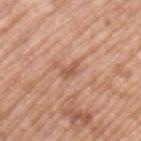biopsy status=total-body-photography surveillance lesion; no biopsy | image source=total-body-photography crop, ~15 mm field of view | lesion diameter=~2.5 mm (longest diameter) | subject=male, about 50 years old | site=the back | tile lighting=white-light.